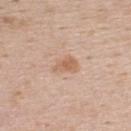No biopsy was performed on this lesion — it was imaged during a full skin examination and was not determined to be concerning. A 15 mm close-up tile from a total-body photography series done for melanoma screening. The patient is a male aged 38 to 42. Longest diameter approximately 3 mm. This is a white-light tile. The lesion-visualizer software estimated a footprint of about 5 mm² and a symmetry-axis asymmetry near 0.35. The lesion is on the upper back.